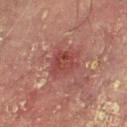Impression: Imaged during a routine full-body skin examination; the lesion was not biopsied and no histopathology is available. Background: A male patient, aged 68–72. This image is a 15 mm lesion crop taken from a total-body photograph. The lesion-visualizer software estimated an area of roughly 8 mm², an eccentricity of roughly 0.7, and a symmetry-axis asymmetry near 0.25. The software also gave a border-irregularity rating of about 3/10 and a within-lesion color-variation index near 4/10. Captured under cross-polarized illumination. Located on the right lower leg. The lesion's longest dimension is about 4 mm.